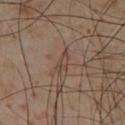diameter — ~3 mm (longest diameter)
patient — male, about 55 years old
imaging modality — ~15 mm crop, total-body skin-cancer survey
image-analysis metrics — an area of roughly 3 mm², a shape eccentricity near 0.9, and two-axis asymmetry of about 0.35
location — the chest
illumination — cross-polarized